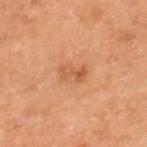Q: How was the tile lit?
A: cross-polarized
Q: Patient demographics?
A: male, roughly 65 years of age
Q: Lesion size?
A: ~3 mm (longest diameter)
Q: What kind of image is this?
A: total-body-photography crop, ~15 mm field of view
Q: Where on the body is the lesion?
A: the leg